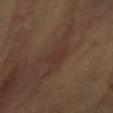– image source · ~15 mm crop, total-body skin-cancer survey
– subject · female, aged 58–62
– anatomic site · the abdomen
– automated metrics · internal color variation of about 1 on a 0–10 scale; a classifier nevus-likeness of about 5/100 and lesion-presence confidence of about 80/100